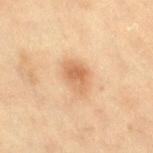follow-up: total-body-photography surveillance lesion; no biopsy
imaging modality: 15 mm crop, total-body photography
automated metrics: a footprint of about 7.5 mm², an eccentricity of roughly 0.75, and two-axis asymmetry of about 0.25; a lesion-to-skin contrast of about 7 (normalized; higher = more distinct); internal color variation of about 4 on a 0–10 scale; a classifier nevus-likeness of about 75/100 and lesion-presence confidence of about 100/100
subject: female, aged 53–57
site: the leg